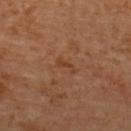Notes:
• workup · imaged on a skin check; not biopsied
• acquisition · ~15 mm crop, total-body skin-cancer survey
• TBP lesion metrics · an average lesion color of about L≈42 a*≈22 b*≈34 (CIELAB), roughly 6 lightness units darker than nearby skin, and a normalized lesion–skin contrast near 5.5; a detector confidence of about 100 out of 100 that the crop contains a lesion
• patient · female, in their mid- to late 50s
• lesion diameter · ~2.5 mm (longest diameter)
• site · the upper back